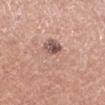image source: total-body-photography crop, ~15 mm field of view | tile lighting: white-light illumination | patient: male, about 55 years old | automated metrics: a footprint of about 11 mm², an outline eccentricity of about 0.35 (0 = round, 1 = elongated), and two-axis asymmetry of about 0.45; border irregularity of about 6.5 on a 0–10 scale, a color-variation rating of about 9/10, and a peripheral color-asymmetry measure near 3.5; a nevus-likeness score of about 20/100 and a lesion-detection confidence of about 100/100 | size: ≈4 mm | body site: the left lower leg.